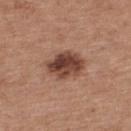Findings:
– notes — total-body-photography surveillance lesion; no biopsy
– patient — female, aged 38–42
– size — ~4.5 mm (longest diameter)
– body site — the back
– acquisition — 15 mm crop, total-body photography
– tile lighting — white-light